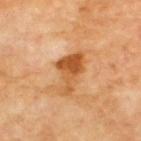Clinical impression:
The lesion was tiled from a total-body skin photograph and was not biopsied.
Image and clinical context:
On the back. Automated tile analysis of the lesion measured a lesion color around L≈54 a*≈26 b*≈43 in CIELAB, roughly 12 lightness units darker than nearby skin, and a normalized border contrast of about 9. And it measured an automated nevus-likeness rating near 20 out of 100 and lesion-presence confidence of about 100/100. Captured under cross-polarized illumination. Longest diameter approximately 5 mm. A male subject, in their 70s. A 15 mm close-up tile from a total-body photography series done for melanoma screening.